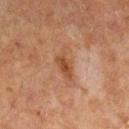Captured during whole-body skin photography for melanoma surveillance; the lesion was not biopsied. From the left lower leg. The patient is a male approximately 65 years of age. Cropped from a total-body skin-imaging series; the visible field is about 15 mm. The lesion's longest dimension is about 4 mm.